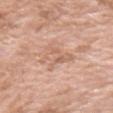Assessment:
Part of a total-body skin-imaging series; this lesion was reviewed on a skin check and was not flagged for biopsy.
Clinical summary:
The total-body-photography lesion software estimated a footprint of about 7 mm² and two-axis asymmetry of about 0.6. The analysis additionally found a lesion color around L≈63 a*≈21 b*≈30 in CIELAB, a lesion–skin lightness drop of about 7, and a normalized lesion–skin contrast near 5. The software also gave border irregularity of about 8.5 on a 0–10 scale, a color-variation rating of about 4/10, and radial color variation of about 1.5. The analysis additionally found an automated nevus-likeness rating near 0 out of 100 and a lesion-detection confidence of about 100/100. A female subject aged approximately 75. The lesion is located on the right upper arm. A roughly 15 mm field-of-view crop from a total-body skin photograph. Approximately 4.5 mm at its widest.